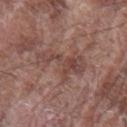Cropped from a whole-body photographic skin survey; the tile spans about 15 mm. The tile uses white-light illumination. The patient is a male roughly 75 years of age. The lesion is located on the arm.